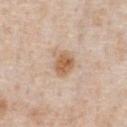| feature | finding |
|---|---|
| biopsy status | imaged on a skin check; not biopsied |
| diameter | ~3 mm (longest diameter) |
| imaging modality | ~15 mm crop, total-body skin-cancer survey |
| lighting | white-light illumination |
| anatomic site | the chest |
| automated lesion analysis | a lesion area of about 6.5 mm², an outline eccentricity of about 0.55 (0 = round, 1 = elongated), and a shape-asymmetry score of about 0.2 (0 = symmetric); a lesion color around L≈61 a*≈18 b*≈34 in CIELAB, about 11 CIELAB-L* units darker than the surrounding skin, and a normalized lesion–skin contrast near 8.5; a border-irregularity index near 2/10 and a color-variation rating of about 3.5/10 |
| subject | male, in their 60s |